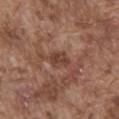biopsy_status: not biopsied; imaged during a skin examination
patient:
  sex: male
  age_approx: 75
lesion_size:
  long_diameter_mm_approx: 2.5
lighting: white-light
image:
  source: total-body photography crop
  field_of_view_mm: 15
automated_metrics:
  vs_skin_darker_L: 9.0
  vs_skin_contrast_norm: 7.0
  border_irregularity_0_10: 2.5
  color_variation_0_10: 2.0
  peripheral_color_asymmetry: 1.0
  nevus_likeness_0_100: 0
  lesion_detection_confidence_0_100: 95
site: abdomen